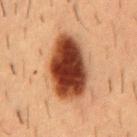This image is a 15 mm lesion crop taken from a total-body photograph. The patient is a male about 55 years old. The lesion is on the mid back.